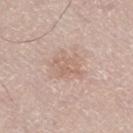Impression:
Imaged during a routine full-body skin examination; the lesion was not biopsied and no histopathology is available.
Acquisition and patient details:
Approximately 4.5 mm at its widest. Cropped from a whole-body photographic skin survey; the tile spans about 15 mm. The subject is a male about 65 years old. The tile uses white-light illumination. An algorithmic analysis of the crop reported a lesion color around L≈64 a*≈17 b*≈26 in CIELAB, a lesion–skin lightness drop of about 7, and a lesion-to-skin contrast of about 4.5 (normalized; higher = more distinct). It also reported border irregularity of about 4 on a 0–10 scale, a color-variation rating of about 2.5/10, and radial color variation of about 1. The lesion is located on the right thigh.